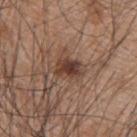biopsy status: total-body-photography surveillance lesion; no biopsy | lesion diameter: ~3.5 mm (longest diameter) | patient: male, about 45 years old | location: the back | illumination: white-light illumination | image-analysis metrics: an eccentricity of roughly 0.75 and a shape-asymmetry score of about 0.25 (0 = symmetric); an average lesion color of about L≈39 a*≈19 b*≈25 (CIELAB) and a normalized lesion–skin contrast near 9.5; lesion-presence confidence of about 100/100 | image: ~15 mm tile from a whole-body skin photo.